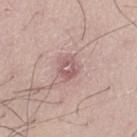Impression:
This lesion was catalogued during total-body skin photography and was not selected for biopsy.
Clinical summary:
Located on the left thigh. The subject is a male roughly 35 years of age. Automated image analysis of the tile measured an outline eccentricity of about 0.55 (0 = round, 1 = elongated) and a symmetry-axis asymmetry near 0.25. And it measured an average lesion color of about L≈58 a*≈21 b*≈20 (CIELAB), about 9 CIELAB-L* units darker than the surrounding skin, and a normalized lesion–skin contrast near 6.5. A 15 mm close-up extracted from a 3D total-body photography capture. This is a white-light tile.